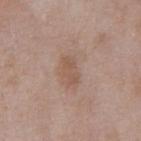Case summary:
* follow-up · imaged on a skin check; not biopsied